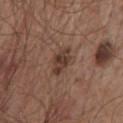The lesion was photographed on a routine skin check and not biopsied; there is no pathology result.
Captured under white-light illumination.
A lesion tile, about 15 mm wide, cut from a 3D total-body photograph.
Located on the front of the torso.
The patient is a male about 65 years old.
Approximately 3.5 mm at its widest.